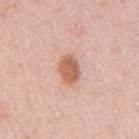• workup · catalogued during a skin exam; not biopsied
• lighting · white-light
• location · the right upper arm
• lesion size · about 3 mm
• acquisition · total-body-photography crop, ~15 mm field of view
• patient · female, aged approximately 30